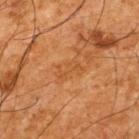Q: Was a biopsy performed?
A: total-body-photography surveillance lesion; no biopsy
Q: How was this image acquired?
A: 15 mm crop, total-body photography
Q: What did automated image analysis measure?
A: a footprint of about 4.5 mm² and a shape eccentricity near 0.85; an average lesion color of about L≈41 a*≈22 b*≈35 (CIELAB), roughly 5 lightness units darker than nearby skin, and a normalized lesion–skin contrast near 4.5; border irregularity of about 6.5 on a 0–10 scale, internal color variation of about 1 on a 0–10 scale, and a peripheral color-asymmetry measure near 0.5; lesion-presence confidence of about 100/100
Q: What are the patient's age and sex?
A: male, aged 63–67
Q: What is the anatomic site?
A: the upper back